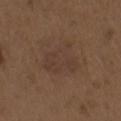Notes:
* follow-up · catalogued during a skin exam; not biopsied
* image source · ~15 mm crop, total-body skin-cancer survey
* subject · male, aged 68 to 72
* anatomic site · the right upper arm